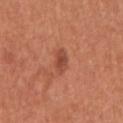biopsy_status: not biopsied; imaged during a skin examination
lesion_size:
  long_diameter_mm_approx: 2.5
image:
  source: total-body photography crop
  field_of_view_mm: 15
patient:
  sex: female
  age_approx: 50
lighting: white-light
site: arm